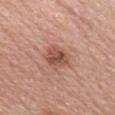Q: What lighting was used for the tile?
A: white-light illumination
Q: What kind of image is this?
A: total-body-photography crop, ~15 mm field of view
Q: Where on the body is the lesion?
A: the chest
Q: Lesion size?
A: ~3.5 mm (longest diameter)
Q: Who is the patient?
A: female, approximately 65 years of age
Q: Automated lesion metrics?
A: a mean CIELAB color near L≈52 a*≈23 b*≈29 and roughly 11 lightness units darker than nearby skin; a border-irregularity rating of about 2/10 and internal color variation of about 5 on a 0–10 scale